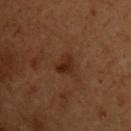{"biopsy_status": "not biopsied; imaged during a skin examination", "automated_metrics": {"border_irregularity_0_10": 3.0}, "patient": {"sex": "male", "age_approx": 50}, "lesion_size": {"long_diameter_mm_approx": 2.5}, "site": "upper back", "image": {"source": "total-body photography crop", "field_of_view_mm": 15}}